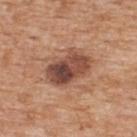workup: total-body-photography surveillance lesion; no biopsy | site: the upper back | image source: 15 mm crop, total-body photography | size: ≈5.5 mm | lighting: white-light illumination | subject: male, roughly 60 years of age | image-analysis metrics: a footprint of about 14 mm²; a border-irregularity rating of about 2/10, a color-variation rating of about 9/10, and radial color variation of about 3; a classifier nevus-likeness of about 30/100 and a lesion-detection confidence of about 100/100.